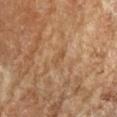Impression: Part of a total-body skin-imaging series; this lesion was reviewed on a skin check and was not flagged for biopsy. Background: A female patient roughly 70 years of age. On the right forearm. A lesion tile, about 15 mm wide, cut from a 3D total-body photograph. Measured at roughly 2.5 mm in maximum diameter.